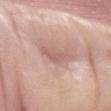biopsy_status: not biopsied; imaged during a skin examination
site: left forearm
image:
  source: total-body photography crop
  field_of_view_mm: 15
patient:
  sex: female
  age_approx: 50
lesion_size:
  long_diameter_mm_approx: 3.5
automated_metrics:
  eccentricity: 0.75
  shape_asymmetry: 0.45
  cielab_L: 60
  cielab_a: 21
  cielab_b: 24
  vs_skin_darker_L: 9.0
  border_irregularity_0_10: 4.5
  color_variation_0_10: 2.0
  lesion_detection_confidence_0_100: 60